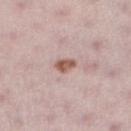workup: imaged on a skin check; not biopsied | illumination: white-light illumination | image-analysis metrics: a lesion area of about 3.5 mm², a shape eccentricity near 0.8, and two-axis asymmetry of about 0.25; a mean CIELAB color near L≈56 a*≈21 b*≈25, roughly 14 lightness units darker than nearby skin, and a normalized border contrast of about 9.5; a nevus-likeness score of about 85/100 and a detector confidence of about 100 out of 100 that the crop contains a lesion | body site: the leg | patient: female, aged 28–32 | image: total-body-photography crop, ~15 mm field of view.